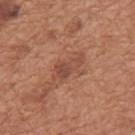Case summary:
– follow-up — imaged on a skin check; not biopsied
– imaging modality — total-body-photography crop, ~15 mm field of view
– site — the back
– patient — male, in their mid-60s
– lesion size — about 4.5 mm